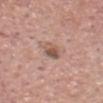This lesion was catalogued during total-body skin photography and was not selected for biopsy.
The tile uses white-light illumination.
The subject is a male in their mid-70s.
A 15 mm crop from a total-body photograph taken for skin-cancer surveillance.
The lesion is on the head or neck.
An algorithmic analysis of the crop reported a mean CIELAB color near L≈54 a*≈19 b*≈26 and roughly 11 lightness units darker than nearby skin.